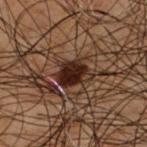Notes:
* notes: no biopsy performed (imaged during a skin exam)
* subject: male, aged 48 to 52
* lighting: cross-polarized
* body site: the mid back
* imaging modality: total-body-photography crop, ~15 mm field of view